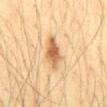Q: Was a biopsy performed?
A: total-body-photography surveillance lesion; no biopsy
Q: Who is the patient?
A: male, aged 33–37
Q: What is the lesion's diameter?
A: ~4.5 mm (longest diameter)
Q: How was the tile lit?
A: cross-polarized illumination
Q: Where on the body is the lesion?
A: the abdomen
Q: What is the imaging modality?
A: ~15 mm crop, total-body skin-cancer survey